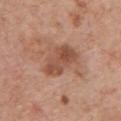notes: no biopsy performed (imaged during a skin exam) | image: ~15 mm crop, total-body skin-cancer survey | illumination: white-light illumination | size: about 4.5 mm | automated metrics: a lesion area of about 10 mm², an eccentricity of roughly 0.8, and a shape-asymmetry score of about 0.25 (0 = symmetric); a detector confidence of about 100 out of 100 that the crop contains a lesion | patient: male, in their 80s | location: the chest.